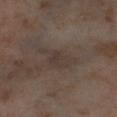Part of a total-body skin-imaging series; this lesion was reviewed on a skin check and was not flagged for biopsy. From the left thigh. A roughly 15 mm field-of-view crop from a total-body skin photograph. Captured under cross-polarized illumination. A female subject, aged 53 to 57. Measured at roughly 4.5 mm in maximum diameter.